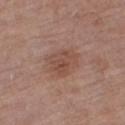{
  "biopsy_status": "not biopsied; imaged during a skin examination",
  "image": {
    "source": "total-body photography crop",
    "field_of_view_mm": 15
  },
  "patient": {
    "sex": "male",
    "age_approx": "85+"
  },
  "automated_metrics": {
    "area_mm2_approx": 9.5,
    "eccentricity": 0.75,
    "shape_asymmetry": 0.25,
    "border_irregularity_0_10": 3.0,
    "color_variation_0_10": 3.5,
    "nevus_likeness_0_100": 15
  },
  "site": "right thigh",
  "lesion_size": {
    "long_diameter_mm_approx": 4.5
  },
  "lighting": "white-light"
}